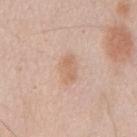Clinical impression: Captured during whole-body skin photography for melanoma surveillance; the lesion was not biopsied. Background: From the chest. The tile uses white-light illumination. The lesion-visualizer software estimated a shape eccentricity near 0.8 and two-axis asymmetry of about 0.15. It also reported a border-irregularity rating of about 1.5/10 and a within-lesion color-variation index near 2/10. Longest diameter approximately 3.5 mm. A close-up tile cropped from a whole-body skin photograph, about 15 mm across. A male patient, approximately 45 years of age.